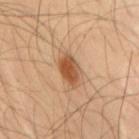<record>
<biopsy_status>not biopsied; imaged during a skin examination</biopsy_status>
</record>